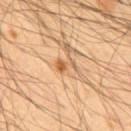Clinical impression: The lesion was tiled from a total-body skin photograph and was not biopsied. Context: The lesion is located on the back. Captured under cross-polarized illumination. Cropped from a whole-body photographic skin survey; the tile spans about 15 mm. About 2.5 mm across. A male subject in their mid- to late 50s.